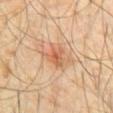{
  "biopsy_status": "not biopsied; imaged during a skin examination",
  "automated_metrics": {
    "cielab_L": 61,
    "cielab_a": 23,
    "cielab_b": 35,
    "vs_skin_darker_L": 9.0,
    "vs_skin_contrast_norm": 6.0,
    "nevus_likeness_0_100": 85,
    "lesion_detection_confidence_0_100": 100
  },
  "image": {
    "source": "total-body photography crop",
    "field_of_view_mm": 15
  },
  "lesion_size": {
    "long_diameter_mm_approx": 3.5
  },
  "patient": {
    "sex": "male",
    "age_approx": 55
  },
  "site": "chest"
}